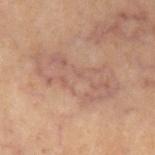The lesion was photographed on a routine skin check and not biopsied; there is no pathology result.